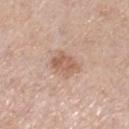Clinical impression: This lesion was catalogued during total-body skin photography and was not selected for biopsy. Background: Captured under white-light illumination. The lesion is located on the right lower leg. This image is a 15 mm lesion crop taken from a total-body photograph. Automated image analysis of the tile measured a lesion area of about 6.5 mm², a shape eccentricity near 0.65, and a symmetry-axis asymmetry near 0.35. The software also gave a mean CIELAB color near L≈59 a*≈20 b*≈29. The analysis additionally found internal color variation of about 2.5 on a 0–10 scale and radial color variation of about 1. The software also gave a classifier nevus-likeness of about 30/100. The lesion's longest dimension is about 3.5 mm. A female patient, approximately 65 years of age.